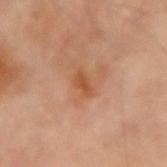{
  "patient": {
    "sex": "male",
    "age_approx": 70
  },
  "site": "right forearm",
  "lesion_size": {
    "long_diameter_mm_approx": 2.5
  },
  "automated_metrics": {
    "area_mm2_approx": 3.0,
    "shape_asymmetry": 0.3,
    "cielab_L": 50,
    "cielab_a": 24,
    "cielab_b": 36,
    "vs_skin_contrast_norm": 7.0,
    "border_irregularity_0_10": 3.0,
    "color_variation_0_10": 1.0,
    "nevus_likeness_0_100": 0,
    "lesion_detection_confidence_0_100": 100
  },
  "image": {
    "source": "total-body photography crop",
    "field_of_view_mm": 15
  }
}